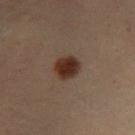follow-up — imaged on a skin check; not biopsied | lighting — cross-polarized | body site — the right lower leg | automated lesion analysis — an average lesion color of about L≈24 a*≈15 b*≈20 (CIELAB), roughly 11 lightness units darker than nearby skin, and a lesion-to-skin contrast of about 12 (normalized; higher = more distinct); lesion-presence confidence of about 100/100 | imaging modality — 15 mm crop, total-body photography | subject — male, in their mid- to late 50s.